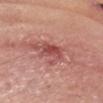Q: Was this lesion biopsied?
A: imaged on a skin check; not biopsied
Q: Where on the body is the lesion?
A: the head or neck
Q: Who is the patient?
A: male, aged 63–67
Q: How was this image acquired?
A: ~15 mm tile from a whole-body skin photo
Q: Illumination type?
A: white-light illumination
Q: How large is the lesion?
A: about 4 mm